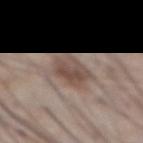Captured during whole-body skin photography for melanoma surveillance; the lesion was not biopsied. A 15 mm close-up extracted from a 3D total-body photography capture. The lesion-visualizer software estimated an average lesion color of about L≈48 a*≈15 b*≈22 (CIELAB) and roughly 10 lightness units darker than nearby skin. It also reported a border-irregularity index near 2.5/10 and a within-lesion color-variation index near 4.5/10. From the abdomen. About 3.5 mm across. A male subject in their mid-50s. The tile uses white-light illumination.